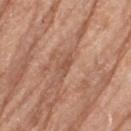– biopsy status: total-body-photography surveillance lesion; no biopsy
– site: the left thigh
– size: ≈2.5 mm
– subject: female, aged 73–77
– image: ~15 mm tile from a whole-body skin photo
– lighting: white-light